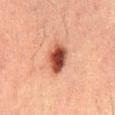A male subject, in their 50s. The lesion is on the mid back. This image is a 15 mm lesion crop taken from a total-body photograph.